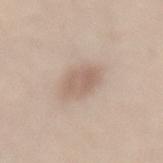Q: Was a biopsy performed?
A: total-body-photography surveillance lesion; no biopsy
Q: Illumination type?
A: white-light illumination
Q: What are the patient's age and sex?
A: female, aged 38 to 42
Q: What is the imaging modality?
A: ~15 mm crop, total-body skin-cancer survey
Q: How large is the lesion?
A: ≈3 mm
Q: What is the anatomic site?
A: the lower back
Q: What did automated image analysis measure?
A: a footprint of about 7 mm², an outline eccentricity of about 0.6 (0 = round, 1 = elongated), and a shape-asymmetry score of about 0.2 (0 = symmetric); a mean CIELAB color near L≈61 a*≈16 b*≈25 and a normalized border contrast of about 6; a border-irregularity rating of about 2/10 and a peripheral color-asymmetry measure near 1